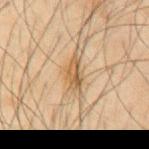Case summary:
– notes · no biopsy performed (imaged during a skin exam)
– diameter · ≈3.5 mm
– imaging modality · ~15 mm crop, total-body skin-cancer survey
– patient · male, in their mid-60s
– illumination · cross-polarized illumination
– location · the chest